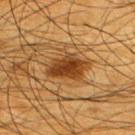Recorded during total-body skin imaging; not selected for excision or biopsy.